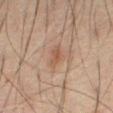Notes:
• biopsy status · catalogued during a skin exam; not biopsied